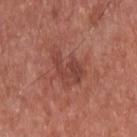notes=no biopsy performed (imaged during a skin exam); image source=total-body-photography crop, ~15 mm field of view; site=the upper back; patient=male, approximately 65 years of age.